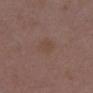Case summary:
– follow-up · catalogued during a skin exam; not biopsied
– anatomic site · the chest
– image · total-body-photography crop, ~15 mm field of view
– patient · female, aged 28–32